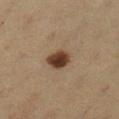workup: imaged on a skin check; not biopsied | imaging modality: 15 mm crop, total-body photography | lesion diameter: about 3 mm | patient: female, roughly 40 years of age | TBP lesion metrics: a shape-asymmetry score of about 0.2 (0 = symmetric); a mean CIELAB color near L≈33 a*≈15 b*≈25 and a lesion-to-skin contrast of about 12.5 (normalized; higher = more distinct) | lighting: cross-polarized | body site: the left lower leg.